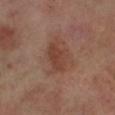Assessment:
The lesion was tiled from a total-body skin photograph and was not biopsied.
Acquisition and patient details:
The patient is a male about 70 years old. A 15 mm crop from a total-body photograph taken for skin-cancer surveillance. The lesion is on the left lower leg.